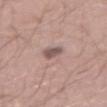Approximately 4.5 mm at its widest. The lesion-visualizer software estimated a border-irregularity index near 3.5/10, internal color variation of about 6 on a 0–10 scale, and radial color variation of about 2. It also reported a nevus-likeness score of about 0/100. A male subject approximately 40 years of age. Located on the back. The tile uses white-light illumination. Cropped from a total-body skin-imaging series; the visible field is about 15 mm.